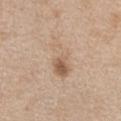workup: imaged on a skin check; not biopsied | subject: female, aged 43 to 47 | lighting: white-light | diameter: ≈5.5 mm | site: the chest | acquisition: total-body-photography crop, ~15 mm field of view | TBP lesion metrics: a mean CIELAB color near L≈61 a*≈16 b*≈30 and about 8 CIELAB-L* units darker than the surrounding skin; an automated nevus-likeness rating near 35 out of 100 and a detector confidence of about 100 out of 100 that the crop contains a lesion.